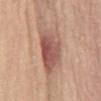{"biopsy_status": "not biopsied; imaged during a skin examination"}